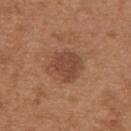Part of a total-body skin-imaging series; this lesion was reviewed on a skin check and was not flagged for biopsy.
The lesion is on the upper back.
A female subject, aged 38–42.
The total-body-photography lesion software estimated roughly 9 lightness units darker than nearby skin and a normalized border contrast of about 6.5. And it measured border irregularity of about 2.5 on a 0–10 scale, internal color variation of about 2 on a 0–10 scale, and a peripheral color-asymmetry measure near 1. It also reported a classifier nevus-likeness of about 15/100 and a lesion-detection confidence of about 100/100.
A lesion tile, about 15 mm wide, cut from a 3D total-body photograph.
Measured at roughly 4 mm in maximum diameter.